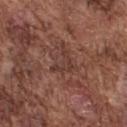<lesion>
<biopsy_status>not biopsied; imaged during a skin examination</biopsy_status>
<lesion_size>
  <long_diameter_mm_approx>3.0</long_diameter_mm_approx>
</lesion_size>
<lighting>white-light</lighting>
<site>upper back</site>
<patient>
  <sex>male</sex>
  <age_approx>75</age_approx>
</patient>
<image>
  <source>total-body photography crop</source>
  <field_of_view_mm>15</field_of_view_mm>
</image>
</lesion>